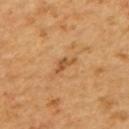| feature | finding |
|---|---|
| follow-up | no biopsy performed (imaged during a skin exam) |
| image source | 15 mm crop, total-body photography |
| patient | male, approximately 60 years of age |
| TBP lesion metrics | a footprint of about 2.5 mm² and a symmetry-axis asymmetry near 0.55 |
| lighting | cross-polarized |
| location | the upper back |
| lesion size | about 3 mm |